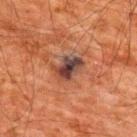Impression:
The lesion was tiled from a total-body skin photograph and was not biopsied.
Background:
The lesion is on the upper back. The patient is a male approximately 60 years of age. A close-up tile cropped from a whole-body skin photograph, about 15 mm across.